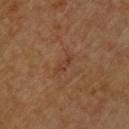Captured during whole-body skin photography for melanoma surveillance; the lesion was not biopsied.
Located on the back.
This image is a 15 mm lesion crop taken from a total-body photograph.
A patient about 60 years old.
The tile uses cross-polarized illumination.